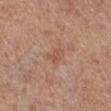Q: Was a biopsy performed?
A: total-body-photography surveillance lesion; no biopsy
Q: What is the anatomic site?
A: the right lower leg
Q: What kind of image is this?
A: ~15 mm crop, total-body skin-cancer survey
Q: What are the patient's age and sex?
A: male, aged 73–77